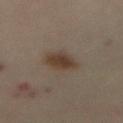Context:
A female patient in their mid- to late 60s. A 15 mm close-up extracted from a 3D total-body photography capture. The lesion is located on the abdomen.